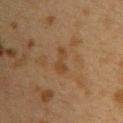follow-up=catalogued during a skin exam; not biopsied
acquisition=~15 mm crop, total-body skin-cancer survey
site=the upper back
patient=female, aged around 40
tile lighting=cross-polarized illumination
lesion diameter=~3.5 mm (longest diameter)